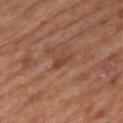Background:
A male patient in their 60s. Cropped from a whole-body photographic skin survey; the tile spans about 15 mm. Approximately 2.5 mm at its widest. This is a cross-polarized tile. The lesion is on the right thigh.